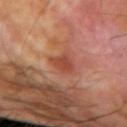Clinical impression: No biopsy was performed on this lesion — it was imaged during a full skin examination and was not determined to be concerning. Clinical summary: On the left forearm. A male patient aged around 70. A 15 mm close-up tile from a total-body photography series done for melanoma screening.